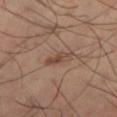Captured during whole-body skin photography for melanoma surveillance; the lesion was not biopsied. Cropped from a total-body skin-imaging series; the visible field is about 15 mm. The total-body-photography lesion software estimated a footprint of about 4 mm². And it measured a peripheral color-asymmetry measure near 0. The patient is a male about 50 years old. This is a cross-polarized tile. The lesion's longest dimension is about 3.5 mm. From the leg.